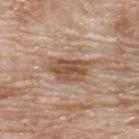{
  "biopsy_status": "not biopsied; imaged during a skin examination",
  "site": "upper back",
  "lesion_size": {
    "long_diameter_mm_approx": 5.0
  },
  "patient": {
    "sex": "male",
    "age_approx": 80
  },
  "image": {
    "source": "total-body photography crop",
    "field_of_view_mm": 15
  },
  "lighting": "white-light"
}